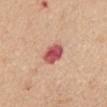The lesion was photographed on a routine skin check and not biopsied; there is no pathology result.
Automated image analysis of the tile measured an average lesion color of about L≈55 a*≈33 b*≈26 (CIELAB), a lesion–skin lightness drop of about 16, and a normalized border contrast of about 11. The analysis additionally found border irregularity of about 2 on a 0–10 scale, a within-lesion color-variation index near 4/10, and a peripheral color-asymmetry measure near 1.
A female subject aged around 40.
About 3 mm across.
Imaged with white-light lighting.
Cropped from a total-body skin-imaging series; the visible field is about 15 mm.
Located on the mid back.